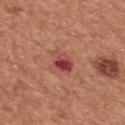| key | value |
|---|---|
| notes | total-body-photography surveillance lesion; no biopsy |
| image | 15 mm crop, total-body photography |
| site | the back |
| subject | male, aged 63 to 67 |
| automated lesion analysis | a lesion area of about 6 mm² and two-axis asymmetry of about 0.3; a border-irregularity index near 3/10, a color-variation rating of about 9/10, and peripheral color asymmetry of about 3; an automated nevus-likeness rating near 0 out of 100 and lesion-presence confidence of about 100/100 |